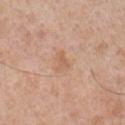<tbp_lesion>
  <automated_metrics>
    <vs_skin_darker_L>7.0</vs_skin_darker_L>
    <vs_skin_contrast_norm>5.0</vs_skin_contrast_norm>
    <border_irregularity_0_10>4.5</border_irregularity_0_10>
    <color_variation_0_10>1.0</color_variation_0_10>
    <peripheral_color_asymmetry>0.5</peripheral_color_asymmetry>
    <nevus_likeness_0_100>0</nevus_likeness_0_100>
    <lesion_detection_confidence_0_100>100</lesion_detection_confidence_0_100>
  </automated_metrics>
  <lesion_size>
    <long_diameter_mm_approx>2.5</long_diameter_mm_approx>
  </lesion_size>
  <lighting>white-light</lighting>
  <site>right upper arm</site>
  <image>
    <source>total-body photography crop</source>
    <field_of_view_mm>15</field_of_view_mm>
  </image>
  <patient>
    <sex>male</sex>
    <age_approx>50</age_approx>
  </patient>
</tbp_lesion>